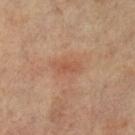Case summary:
* follow-up · no biopsy performed (imaged during a skin exam)
* illumination · cross-polarized illumination
* anatomic site · the right leg
* subject · female, aged 63 to 67
* lesion size · about 3.5 mm
* image source · total-body-photography crop, ~15 mm field of view